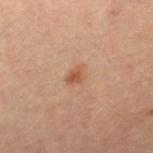The lesion was photographed on a routine skin check and not biopsied; there is no pathology result. The recorded lesion diameter is about 2.5 mm. Automated tile analysis of the lesion measured border irregularity of about 3 on a 0–10 scale and a color-variation rating of about 2/10. It also reported an automated nevus-likeness rating near 55 out of 100. A female subject, aged around 45. On the leg. A lesion tile, about 15 mm wide, cut from a 3D total-body photograph.